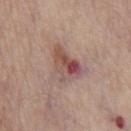  biopsy_status: not biopsied; imaged during a skin examination
  image:
    source: total-body photography crop
    field_of_view_mm: 15
  site: chest
  patient:
    sex: male
    age_approx: 65